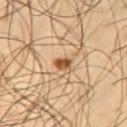<lesion>
  <biopsy_status>not biopsied; imaged during a skin examination</biopsy_status>
  <site>leg</site>
  <patient>
    <sex>male</sex>
    <age_approx>45</age_approx>
  </patient>
  <lesion_size>
    <long_diameter_mm_approx>2.5</long_diameter_mm_approx>
  </lesion_size>
  <lighting>cross-polarized</lighting>
  <image>
    <source>total-body photography crop</source>
    <field_of_view_mm>15</field_of_view_mm>
  </image>
</lesion>